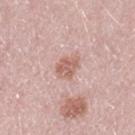notes — total-body-photography surveillance lesion; no biopsy